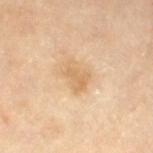Case summary:
- follow-up · no biopsy performed (imaged during a skin exam)
- tile lighting · cross-polarized
- image source · total-body-photography crop, ~15 mm field of view
- lesion size · ≈3.5 mm
- patient · female, in their mid-70s
- site · the right thigh
- automated lesion analysis · an eccentricity of roughly 0.75 and a shape-asymmetry score of about 0.35 (0 = symmetric); a mean CIELAB color near L≈68 a*≈17 b*≈39 and a normalized border contrast of about 6